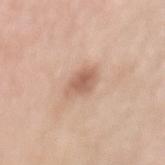Imaged during a routine full-body skin examination; the lesion was not biopsied and no histopathology is available. Measured at roughly 3.5 mm in maximum diameter. On the mid back. A roughly 15 mm field-of-view crop from a total-body skin photograph. An algorithmic analysis of the crop reported roughly 11 lightness units darker than nearby skin and a lesion-to-skin contrast of about 7.5 (normalized; higher = more distinct). The subject is a female approximately 70 years of age.